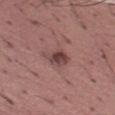Q: Is there a histopathology result?
A: no biopsy performed (imaged during a skin exam)
Q: Lesion location?
A: the left thigh
Q: What is the imaging modality?
A: ~15 mm tile from a whole-body skin photo
Q: What are the patient's age and sex?
A: male, in their mid- to late 40s